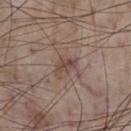The lesion was photographed on a routine skin check and not biopsied; there is no pathology result. The lesion-visualizer software estimated an average lesion color of about L≈45 a*≈18 b*≈23 (CIELAB) and a lesion-to-skin contrast of about 6.5 (normalized; higher = more distinct). The software also gave a border-irregularity index near 3/10. The software also gave a lesion-detection confidence of about 100/100. Longest diameter approximately 2.5 mm. A region of skin cropped from a whole-body photographic capture, roughly 15 mm wide. The patient is a male aged approximately 75. On the chest.